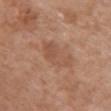workup: catalogued during a skin exam; not biopsied
subject: female, in their 70s
body site: the front of the torso
imaging modality: total-body-photography crop, ~15 mm field of view
illumination: white-light illumination
lesion diameter: ~5 mm (longest diameter)